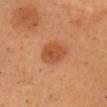follow-up: total-body-photography surveillance lesion; no biopsy | image source: ~15 mm crop, total-body skin-cancer survey | patient: male, aged 38 to 42 | anatomic site: the head or neck | automated metrics: a mean CIELAB color near L≈45 a*≈25 b*≈34, a lesion–skin lightness drop of about 9, and a normalized border contrast of about 7; a color-variation rating of about 2.5/10 and a peripheral color-asymmetry measure near 1.